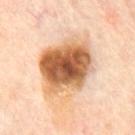Impression: The lesion was tiled from a total-body skin photograph and was not biopsied.